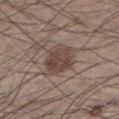Recorded during total-body skin imaging; not selected for excision or biopsy.
Measured at roughly 4.5 mm in maximum diameter.
From the left lower leg.
A 15 mm close-up extracted from a 3D total-body photography capture.
Captured under white-light illumination.
A male patient aged 33–37.